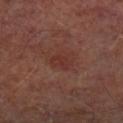Part of a total-body skin-imaging series; this lesion was reviewed on a skin check and was not flagged for biopsy.
The recorded lesion diameter is about 3 mm.
Located on the left lower leg.
Imaged with cross-polarized lighting.
Automated image analysis of the tile measured an area of roughly 4.5 mm², an outline eccentricity of about 0.75 (0 = round, 1 = elongated), and a symmetry-axis asymmetry near 0.25. It also reported a detector confidence of about 100 out of 100 that the crop contains a lesion.
The subject is a male in their mid-60s.
A close-up tile cropped from a whole-body skin photograph, about 15 mm across.